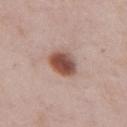Imaged during a routine full-body skin examination; the lesion was not biopsied and no histopathology is available. The lesion is located on the chest. The total-body-photography lesion software estimated a footprint of about 8.5 mm² and an eccentricity of roughly 0.4. And it measured a classifier nevus-likeness of about 100/100 and a lesion-detection confidence of about 100/100. Captured under white-light illumination. The subject is a male aged approximately 55. A 15 mm crop from a total-body photograph taken for skin-cancer surveillance.